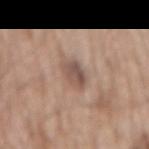Imaged during a routine full-body skin examination; the lesion was not biopsied and no histopathology is available. Longest diameter approximately 3 mm. Captured under white-light illumination. A male subject approximately 60 years of age. A roughly 15 mm field-of-view crop from a total-body skin photograph. The lesion is on the front of the torso.